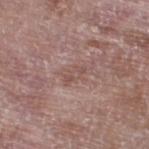follow-up: total-body-photography surveillance lesion; no biopsy
subject: male, aged around 75
image: 15 mm crop, total-body photography
lighting: white-light
site: the left lower leg